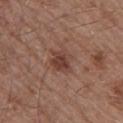{"biopsy_status": "not biopsied; imaged during a skin examination", "automated_metrics": {"vs_skin_contrast_norm": 8.0, "border_irregularity_0_10": 2.5, "color_variation_0_10": 2.5, "peripheral_color_asymmetry": 1.0}, "patient": {"sex": "male", "age_approx": 55}, "lesion_size": {"long_diameter_mm_approx": 3.0}, "lighting": "white-light", "image": {"source": "total-body photography crop", "field_of_view_mm": 15}, "site": "upper back"}